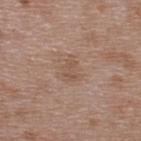– follow-up: total-body-photography surveillance lesion; no biopsy
– automated metrics: an area of roughly 3 mm² and a shape-asymmetry score of about 0.45 (0 = symmetric); border irregularity of about 5.5 on a 0–10 scale, internal color variation of about 0 on a 0–10 scale, and radial color variation of about 0; an automated nevus-likeness rating near 0 out of 100 and lesion-presence confidence of about 100/100
– illumination: white-light illumination
– subject: male, about 50 years old
– lesion diameter: ≈2.5 mm
– image source: total-body-photography crop, ~15 mm field of view
– anatomic site: the upper back